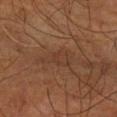biopsy status: catalogued during a skin exam; not biopsied
location: the arm
imaging modality: total-body-photography crop, ~15 mm field of view
patient: male, approximately 60 years of age
tile lighting: cross-polarized illumination
image-analysis metrics: a footprint of about 7 mm² and a shape-asymmetry score of about 0.45 (0 = symmetric)